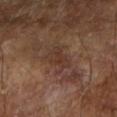Clinical impression: No biopsy was performed on this lesion — it was imaged during a full skin examination and was not determined to be concerning. Background: The subject is a male in their mid- to late 60s. The lesion is located on the right forearm. Measured at roughly 4 mm in maximum diameter. Cropped from a total-body skin-imaging series; the visible field is about 15 mm.